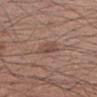{
  "biopsy_status": "not biopsied; imaged during a skin examination",
  "image": {
    "source": "total-body photography crop",
    "field_of_view_mm": 15
  },
  "patient": {
    "sex": "male",
    "age_approx": 60
  },
  "lesion_size": {
    "long_diameter_mm_approx": 3.0
  },
  "site": "right upper arm",
  "lighting": "white-light"
}